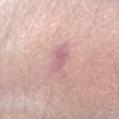Imaged during a routine full-body skin examination; the lesion was not biopsied and no histopathology is available.
Automated tile analysis of the lesion measured a lesion area of about 4 mm² and two-axis asymmetry of about 0.25. The analysis additionally found a mean CIELAB color near L≈63 a*≈24 b*≈18, about 9 CIELAB-L* units darker than the surrounding skin, and a normalized lesion–skin contrast near 6. The software also gave a border-irregularity rating of about 2.5/10 and a peripheral color-asymmetry measure near 1. It also reported a classifier nevus-likeness of about 0/100 and lesion-presence confidence of about 75/100.
Imaged with white-light lighting.
On the left forearm.
This image is a 15 mm lesion crop taken from a total-body photograph.
A female subject aged 63 to 67.